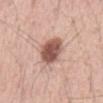<case>
<biopsy_status>not biopsied; imaged during a skin examination</biopsy_status>
<image>
  <source>total-body photography crop</source>
  <field_of_view_mm>15</field_of_view_mm>
</image>
<site>abdomen</site>
<patient>
  <sex>male</sex>
  <age_approx>55</age_approx>
</patient>
<lesion_size>
  <long_diameter_mm_approx>4.5</long_diameter_mm_approx>
</lesion_size>
</case>